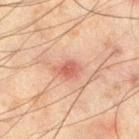The lesion is on the left thigh. A 15 mm crop from a total-body photograph taken for skin-cancer surveillance. The tile uses cross-polarized illumination. The subject is a male in their mid-40s.